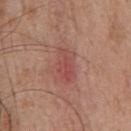Imaged during a routine full-body skin examination; the lesion was not biopsied and no histopathology is available.
A male patient, in their mid- to late 50s.
This image is a 15 mm lesion crop taken from a total-body photograph.
The lesion is on the front of the torso.